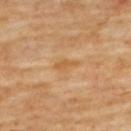<case>
<biopsy_status>not biopsied; imaged during a skin examination</biopsy_status>
<site>upper back</site>
<lighting>cross-polarized</lighting>
<patient>
  <sex>female</sex>
  <age_approx>60</age_approx>
</patient>
<image>
  <source>total-body photography crop</source>
  <field_of_view_mm>15</field_of_view_mm>
</image>
<lesion_size>
  <long_diameter_mm_approx>3.0</long_diameter_mm_approx>
</lesion_size>
</case>